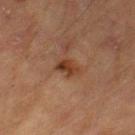follow-up = total-body-photography surveillance lesion; no biopsy
image source = ~15 mm tile from a whole-body skin photo
anatomic site = the left thigh
patient = male, about 85 years old or older